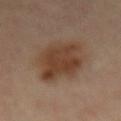Q: How was the tile lit?
A: cross-polarized illumination
Q: How was this image acquired?
A: ~15 mm crop, total-body skin-cancer survey
Q: Who is the patient?
A: male, aged approximately 65
Q: Where on the body is the lesion?
A: the lower back
Q: What is the lesion's diameter?
A: ~7.5 mm (longest diameter)